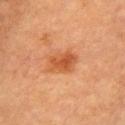This lesion was catalogued during total-body skin photography and was not selected for biopsy. The total-body-photography lesion software estimated border irregularity of about 2.5 on a 0–10 scale, a within-lesion color-variation index near 4/10, and radial color variation of about 1.5. A female subject, aged 58–62. Longest diameter approximately 4.5 mm. This is a cross-polarized tile. The lesion is on the chest. A lesion tile, about 15 mm wide, cut from a 3D total-body photograph.